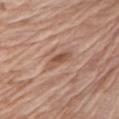{
  "biopsy_status": "not biopsied; imaged during a skin examination",
  "automated_metrics": {
    "area_mm2_approx": 4.0,
    "eccentricity": 0.85,
    "shape_asymmetry": 0.25,
    "cielab_L": 52,
    "cielab_a": 21,
    "cielab_b": 29,
    "vs_skin_darker_L": 10.0,
    "vs_skin_contrast_norm": 7.0,
    "border_irregularity_0_10": 2.5,
    "color_variation_0_10": 3.0
  },
  "lesion_size": {
    "long_diameter_mm_approx": 3.0
  },
  "site": "left upper arm",
  "patient": {
    "sex": "female",
    "age_approx": 75
  },
  "image": {
    "source": "total-body photography crop",
    "field_of_view_mm": 15
  },
  "lighting": "white-light"
}